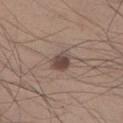{
  "biopsy_status": "not biopsied; imaged during a skin examination",
  "site": "right lower leg",
  "patient": {
    "sex": "male",
    "age_approx": 30
  },
  "lesion_size": {
    "long_diameter_mm_approx": 3.0
  },
  "image": {
    "source": "total-body photography crop",
    "field_of_view_mm": 15
  }
}